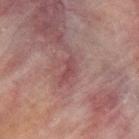Part of a total-body skin-imaging series; this lesion was reviewed on a skin check and was not flagged for biopsy.
Measured at roughly 3 mm in maximum diameter.
This is a cross-polarized tile.
A 15 mm close-up tile from a total-body photography series done for melanoma screening.
An algorithmic analysis of the crop reported a mean CIELAB color near L≈37 a*≈21 b*≈16 and a lesion-to-skin contrast of about 6 (normalized; higher = more distinct).
From the upper back.
The patient is a male approximately 70 years of age.